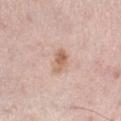notes: imaged on a skin check; not biopsied | image source: 15 mm crop, total-body photography | anatomic site: the right lower leg | patient: female, aged 43 to 47.